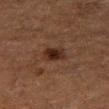Recorded during total-body skin imaging; not selected for excision or biopsy. The patient is a male aged 73 to 77. This image is a 15 mm lesion crop taken from a total-body photograph. Located on the right thigh. Imaged with cross-polarized lighting. An algorithmic analysis of the crop reported two-axis asymmetry of about 0.25. And it measured a border-irregularity index near 2.5/10, internal color variation of about 3.5 on a 0–10 scale, and radial color variation of about 1. And it measured an automated nevus-likeness rating near 90 out of 100 and a lesion-detection confidence of about 100/100.